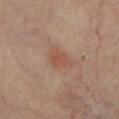Imaged during a routine full-body skin examination; the lesion was not biopsied and no histopathology is available. This is a cross-polarized tile. The lesion's longest dimension is about 3.5 mm. From the left lower leg. The total-body-photography lesion software estimated an average lesion color of about L≈51 a*≈20 b*≈28 (CIELAB) and about 6 CIELAB-L* units darker than the surrounding skin. The software also gave a border-irregularity rating of about 2.5/10, a color-variation rating of about 2.5/10, and radial color variation of about 1. And it measured a nevus-likeness score of about 15/100 and a lesion-detection confidence of about 100/100. A female patient roughly 65 years of age. A region of skin cropped from a whole-body photographic capture, roughly 15 mm wide.